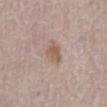Clinical summary:
A close-up tile cropped from a whole-body skin photograph, about 15 mm across. From the abdomen. The recorded lesion diameter is about 3 mm. The tile uses white-light illumination. A female patient in their 80s.